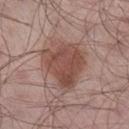Clinical impression:
The lesion was tiled from a total-body skin photograph and was not biopsied.
Clinical summary:
A male subject, aged around 55. On the right lower leg. About 6 mm across. A 15 mm close-up tile from a total-body photography series done for melanoma screening. The tile uses white-light illumination. The lesion-visualizer software estimated a lesion area of about 21 mm² and an outline eccentricity of about 0.55 (0 = round, 1 = elongated). It also reported roughly 11 lightness units darker than nearby skin and a lesion-to-skin contrast of about 8.5 (normalized; higher = more distinct). The software also gave radial color variation of about 1.5. The analysis additionally found a detector confidence of about 100 out of 100 that the crop contains a lesion.